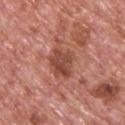This lesion was catalogued during total-body skin photography and was not selected for biopsy. This image is a 15 mm lesion crop taken from a total-body photograph. A male patient, aged 73 to 77. On the upper back.